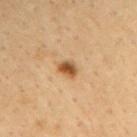Recorded during total-body skin imaging; not selected for excision or biopsy. A close-up tile cropped from a whole-body skin photograph, about 15 mm across. A male subject, aged 63 to 67. Captured under cross-polarized illumination. An algorithmic analysis of the crop reported an eccentricity of roughly 0.65 and two-axis asymmetry of about 0.25. And it measured a peripheral color-asymmetry measure near 1. Located on the right upper arm.